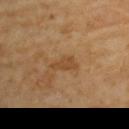Captured during whole-body skin photography for melanoma surveillance; the lesion was not biopsied.
The lesion's longest dimension is about 3.5 mm.
A 15 mm close-up extracted from a 3D total-body photography capture.
A female subject, aged 63–67.
The tile uses cross-polarized illumination.
From the left upper arm.